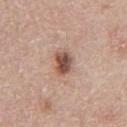acquisition = ~15 mm tile from a whole-body skin photo
lesion size = ~3 mm (longest diameter)
body site = the chest
illumination = white-light
patient = male, aged 63 to 67